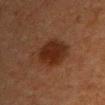{
  "biopsy_status": "not biopsied; imaged during a skin examination",
  "image": {
    "source": "total-body photography crop",
    "field_of_view_mm": 15
  },
  "patient": {
    "sex": "female",
    "age_approx": 55
  },
  "lighting": "cross-polarized",
  "site": "chest",
  "lesion_size": {
    "long_diameter_mm_approx": 4.5
  }
}